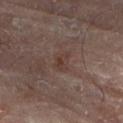The lesion was tiled from a total-body skin photograph and was not biopsied.